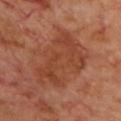Q: Is there a histopathology result?
A: total-body-photography surveillance lesion; no biopsy
Q: What is the anatomic site?
A: the chest
Q: What is the lesion's diameter?
A: ~8 mm (longest diameter)
Q: Who is the patient?
A: male, about 70 years old
Q: Automated lesion metrics?
A: an area of roughly 28 mm² and a symmetry-axis asymmetry near 0.35; a color-variation rating of about 3/10 and peripheral color asymmetry of about 1; a nevus-likeness score of about 0/100
Q: What kind of image is this?
A: ~15 mm crop, total-body skin-cancer survey
Q: Illumination type?
A: cross-polarized